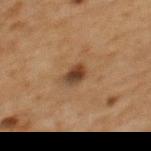follow-up=total-body-photography surveillance lesion; no biopsy
image=total-body-photography crop, ~15 mm field of view
location=the back
illumination=cross-polarized illumination
patient=female, aged around 60
size=≈2.5 mm